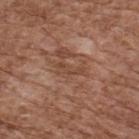Q: Was this lesion biopsied?
A: total-body-photography surveillance lesion; no biopsy
Q: What is the lesion's diameter?
A: ~3.5 mm (longest diameter)
Q: Automated lesion metrics?
A: an area of roughly 3.5 mm², an outline eccentricity of about 0.95 (0 = round, 1 = elongated), and two-axis asymmetry of about 0.6; a nevus-likeness score of about 0/100 and a lesion-detection confidence of about 70/100
Q: Who is the patient?
A: male, in their mid-70s
Q: Lesion location?
A: the upper back
Q: How was the tile lit?
A: white-light illumination
Q: How was this image acquired?
A: ~15 mm tile from a whole-body skin photo